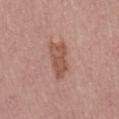{
  "biopsy_status": "not biopsied; imaged during a skin examination",
  "patient": {
    "sex": "male",
    "age_approx": 40
  },
  "lighting": "white-light",
  "site": "mid back",
  "lesion_size": {
    "long_diameter_mm_approx": 4.5
  },
  "image": {
    "source": "total-body photography crop",
    "field_of_view_mm": 15
  }
}